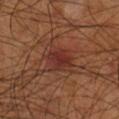Context: The tile uses cross-polarized illumination. An algorithmic analysis of the crop reported border irregularity of about 2 on a 0–10 scale, a within-lesion color-variation index near 4/10, and a peripheral color-asymmetry measure near 1.5. The lesion's longest dimension is about 3.5 mm. A lesion tile, about 15 mm wide, cut from a 3D total-body photograph. On the right lower leg. A male subject, roughly 70 years of age.